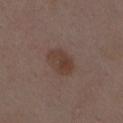| field | value |
|---|---|
| notes | no biopsy performed (imaged during a skin exam) |
| image-analysis metrics | an average lesion color of about L≈39 a*≈16 b*≈23 (CIELAB), roughly 7 lightness units darker than nearby skin, and a normalized border contrast of about 7; peripheral color asymmetry of about 1; an automated nevus-likeness rating near 55 out of 100 |
| patient | female, about 30 years old |
| lesion size | ≈4 mm |
| lighting | white-light illumination |
| location | the back |
| image source | ~15 mm crop, total-body skin-cancer survey |